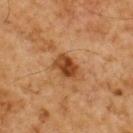– follow-up · catalogued during a skin exam; not biopsied
– lesion diameter · ≈3 mm
– subject · male, roughly 60 years of age
– image · 15 mm crop, total-body photography
– lighting · cross-polarized illumination
– location · the upper back